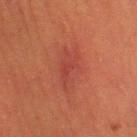  biopsy_status: not biopsied; imaged during a skin examination
  lighting: cross-polarized
  image:
    source: total-body photography crop
    field_of_view_mm: 15
  automated_metrics:
    cielab_L: 37
    cielab_a: 28
    cielab_b: 26
    vs_skin_darker_L: 5.0
    vs_skin_contrast_norm: 5.0
    border_irregularity_0_10: 3.0
    peripheral_color_asymmetry: 1.0
  patient:
    sex: male
    age_approx: 40
  site: left lower leg
  lesion_size:
    long_diameter_mm_approx: 5.5